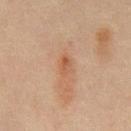This lesion was catalogued during total-body skin photography and was not selected for biopsy.
The lesion's longest dimension is about 2.5 mm.
Cropped from a whole-body photographic skin survey; the tile spans about 15 mm.
A male patient, roughly 45 years of age.
Located on the abdomen.